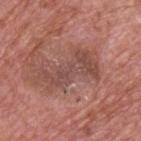Q: What is the lesion's diameter?
A: ~7 mm (longest diameter)
Q: What lighting was used for the tile?
A: white-light
Q: What is the anatomic site?
A: the upper back
Q: Who is the patient?
A: male, approximately 75 years of age
Q: What kind of image is this?
A: 15 mm crop, total-body photography